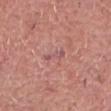Q: Was a biopsy performed?
A: no biopsy performed (imaged during a skin exam)
Q: What lighting was used for the tile?
A: white-light illumination
Q: What is the anatomic site?
A: the head or neck
Q: How large is the lesion?
A: ≈3 mm
Q: Patient demographics?
A: male, aged 58 to 62
Q: Automated lesion metrics?
A: an area of roughly 3 mm² and an outline eccentricity of about 0.9 (0 = round, 1 = elongated); a border-irregularity rating of about 4/10 and a color-variation rating of about 0/10; an automated nevus-likeness rating near 0 out of 100 and a lesion-detection confidence of about 90/100
Q: How was this image acquired?
A: total-body-photography crop, ~15 mm field of view